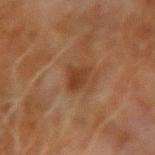Q: Was this lesion biopsied?
A: imaged on a skin check; not biopsied
Q: What is the anatomic site?
A: the right upper arm
Q: Who is the patient?
A: male, aged 68 to 72
Q: What kind of image is this?
A: ~15 mm tile from a whole-body skin photo